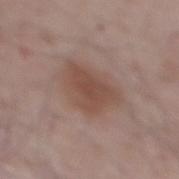notes = imaged on a skin check; not biopsied
image = 15 mm crop, total-body photography
lesion diameter = ≈5.5 mm
anatomic site = the mid back
subject = male, approximately 65 years of age
TBP lesion metrics = a classifier nevus-likeness of about 60/100 and a lesion-detection confidence of about 100/100